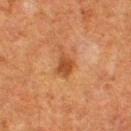Assessment: Part of a total-body skin-imaging series; this lesion was reviewed on a skin check and was not flagged for biopsy. Context: The lesion is located on the right thigh. Imaged with cross-polarized lighting. A lesion tile, about 15 mm wide, cut from a 3D total-body photograph. The total-body-photography lesion software estimated a footprint of about 5 mm². The software also gave internal color variation of about 2.5 on a 0–10 scale. A female subject, aged around 50.